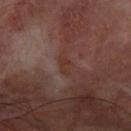The lesion was tiled from a total-body skin photograph and was not biopsied.
A male patient aged 63 to 67.
On the left forearm.
A 15 mm close-up extracted from a 3D total-body photography capture.